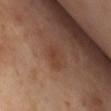Imaged during a routine full-body skin examination; the lesion was not biopsied and no histopathology is available.
Measured at roughly 2.5 mm in maximum diameter.
The subject is a female aged around 55.
This is a cross-polarized tile.
Located on the abdomen.
A region of skin cropped from a whole-body photographic capture, roughly 15 mm wide.